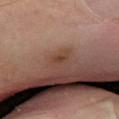Findings:
– notes: total-body-photography surveillance lesion; no biopsy
– location: the left leg
– imaging modality: ~15 mm crop, total-body skin-cancer survey
– subject: male, about 50 years old